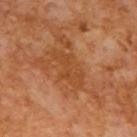Assessment:
Recorded during total-body skin imaging; not selected for excision or biopsy.
Background:
Captured under cross-polarized illumination. A male subject, aged 63 to 67. A lesion tile, about 15 mm wide, cut from a 3D total-body photograph.